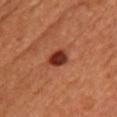No biopsy was performed on this lesion — it was imaged during a full skin examination and was not determined to be concerning. An algorithmic analysis of the crop reported a lesion color around L≈34 a*≈27 b*≈31 in CIELAB and roughly 14 lightness units darker than nearby skin. On the front of the torso. Cropped from a total-body skin-imaging series; the visible field is about 15 mm. The tile uses cross-polarized illumination. A female patient approximately 65 years of age.